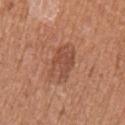  biopsy_status: not biopsied; imaged during a skin examination
  image:
    source: total-body photography crop
    field_of_view_mm: 15
  lighting: white-light
  patient:
    sex: female
    age_approx: 55
  automated_metrics:
    border_irregularity_0_10: 2.5
    peripheral_color_asymmetry: 1.0
  site: left upper arm